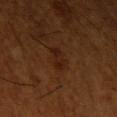<tbp_lesion>
  <biopsy_status>not biopsied; imaged during a skin examination</biopsy_status>
  <lesion_size>
    <long_diameter_mm_approx>2.5</long_diameter_mm_approx>
  </lesion_size>
  <image>
    <source>total-body photography crop</source>
    <field_of_view_mm>15</field_of_view_mm>
  </image>
  <site>arm</site>
  <lighting>cross-polarized</lighting>
  <patient>
    <sex>male</sex>
    <age_approx>65</age_approx>
  </patient>
</tbp_lesion>